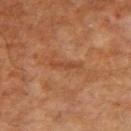This is a cross-polarized tile.
Automated tile analysis of the lesion measured an area of roughly 2.5 mm², an eccentricity of roughly 0.95, and two-axis asymmetry of about 0.45. The analysis additionally found an average lesion color of about L≈44 a*≈24 b*≈35 (CIELAB), a lesion–skin lightness drop of about 7, and a normalized lesion–skin contrast near 5.5. The analysis additionally found a border-irregularity rating of about 6/10, internal color variation of about 0 on a 0–10 scale, and peripheral color asymmetry of about 0. And it measured an automated nevus-likeness rating near 0 out of 100 and a lesion-detection confidence of about 95/100.
A 15 mm close-up tile from a total-body photography series done for melanoma screening.
On the arm.
A male subject approximately 65 years of age.
The recorded lesion diameter is about 3.5 mm.